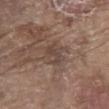workup: catalogued during a skin exam; not biopsied
subject: male, aged 78–82
tile lighting: white-light illumination
image: ~15 mm crop, total-body skin-cancer survey
site: the abdomen